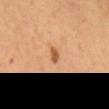{
  "biopsy_status": "not biopsied; imaged during a skin examination",
  "image": {
    "source": "total-body photography crop",
    "field_of_view_mm": 15
  },
  "site": "abdomen",
  "lesion_size": {
    "long_diameter_mm_approx": 2.0
  },
  "automated_metrics": {
    "area_mm2_approx": 2.5,
    "shape_asymmetry": 0.25,
    "nevus_likeness_0_100": 80,
    "lesion_detection_confidence_0_100": 100
  },
  "patient": {
    "sex": "female"
  }
}